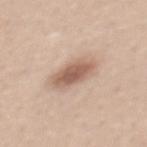Q: Was this lesion biopsied?
A: total-body-photography surveillance lesion; no biopsy
Q: How was the tile lit?
A: white-light illumination
Q: Where on the body is the lesion?
A: the chest
Q: What kind of image is this?
A: 15 mm crop, total-body photography
Q: What are the patient's age and sex?
A: female, aged approximately 25
Q: How large is the lesion?
A: about 4.5 mm